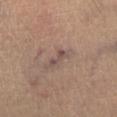Imaged during a routine full-body skin examination; the lesion was not biopsied and no histopathology is available. A lesion tile, about 15 mm wide, cut from a 3D total-body photograph. The subject is a male in their 60s. The tile uses cross-polarized illumination. The lesion is located on the right lower leg. An algorithmic analysis of the crop reported an area of roughly 3.5 mm², an eccentricity of roughly 0.9, and a shape-asymmetry score of about 0.35 (0 = symmetric). The analysis additionally found a lesion color around L≈46 a*≈15 b*≈19 in CIELAB, about 7 CIELAB-L* units darker than the surrounding skin, and a normalized border contrast of about 6.5. Approximately 3 mm at its widest.